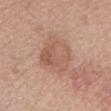Recorded during total-body skin imaging; not selected for excision or biopsy. This is a white-light tile. A female subject in their mid- to late 30s. On the head or neck. A lesion tile, about 15 mm wide, cut from a 3D total-body photograph. Longest diameter approximately 4.5 mm.